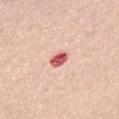biopsy status: catalogued during a skin exam; not biopsied | lighting: white-light illumination | acquisition: ~15 mm tile from a whole-body skin photo | location: the chest | subject: female, aged approximately 65.